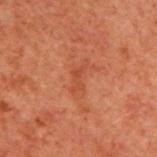The lesion was photographed on a routine skin check and not biopsied; there is no pathology result.